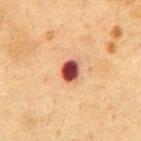Recorded during total-body skin imaging; not selected for excision or biopsy.
This is a cross-polarized tile.
Cropped from a total-body skin-imaging series; the visible field is about 15 mm.
A male patient, aged 73–77.
The lesion is on the abdomen.
The recorded lesion diameter is about 2.5 mm.